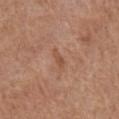Imaged during a routine full-body skin examination; the lesion was not biopsied and no histopathology is available. About 2.5 mm across. The tile uses white-light illumination. An algorithmic analysis of the crop reported a lesion color around L≈51 a*≈22 b*≈31 in CIELAB, a lesion–skin lightness drop of about 7, and a normalized lesion–skin contrast near 5.5. Cropped from a total-body skin-imaging series; the visible field is about 15 mm. On the front of the torso. A male patient aged around 65.